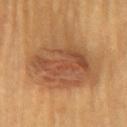Clinical impression:
No biopsy was performed on this lesion — it was imaged during a full skin examination and was not determined to be concerning.
Clinical summary:
Imaged with cross-polarized lighting. The subject is a female in their mid-60s. Located on the chest. The lesion-visualizer software estimated a border-irregularity rating of about 4/10 and radial color variation of about 1.5. The recorded lesion diameter is about 9 mm. A lesion tile, about 15 mm wide, cut from a 3D total-body photograph.